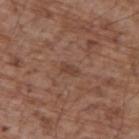The lesion was photographed on a routine skin check and not biopsied; there is no pathology result. The tile uses white-light illumination. A roughly 15 mm field-of-view crop from a total-body skin photograph. From the back. The patient is a male approximately 70 years of age. An algorithmic analysis of the crop reported a lesion area of about 3 mm², an eccentricity of roughly 0.9, and two-axis asymmetry of about 0.25. It also reported a mean CIELAB color near L≈42 a*≈19 b*≈26, roughly 7 lightness units darker than nearby skin, and a lesion-to-skin contrast of about 5.5 (normalized; higher = more distinct). The software also gave internal color variation of about 0.5 on a 0–10 scale and peripheral color asymmetry of about 0. The software also gave a nevus-likeness score of about 0/100 and lesion-presence confidence of about 100/100.